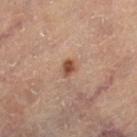Part of a total-body skin-imaging series; this lesion was reviewed on a skin check and was not flagged for biopsy.
The lesion is located on the leg.
About 2 mm across.
A close-up tile cropped from a whole-body skin photograph, about 15 mm across.
A female subject aged 63 to 67.